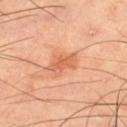The lesion was photographed on a routine skin check and not biopsied; there is no pathology result.
Cropped from a whole-body photographic skin survey; the tile spans about 15 mm.
Approximately 3.5 mm at its widest.
This is a cross-polarized tile.
A male patient about 65 years old.
The lesion is located on the right thigh.
Automated image analysis of the tile measured an area of roughly 7 mm², an outline eccentricity of about 0.7 (0 = round, 1 = elongated), and a shape-asymmetry score of about 0.3 (0 = symmetric). The software also gave a border-irregularity index near 3.5/10, a color-variation rating of about 4/10, and radial color variation of about 1.5. The analysis additionally found a nevus-likeness score of about 30/100.